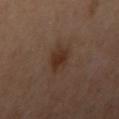- workup: imaged on a skin check; not biopsied
- lesion diameter: ≈3 mm
- site: the left arm
- subject: female, roughly 40 years of age
- image: ~15 mm crop, total-body skin-cancer survey
- tile lighting: cross-polarized illumination